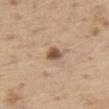Impression: No biopsy was performed on this lesion — it was imaged during a full skin examination and was not determined to be concerning. Clinical summary: Captured under white-light illumination. Cropped from a whole-body photographic skin survey; the tile spans about 15 mm. From the left thigh. Automated image analysis of the tile measured a footprint of about 4 mm², a shape eccentricity near 0.7, and a symmetry-axis asymmetry near 0.2. And it measured a mean CIELAB color near L≈54 a*≈18 b*≈30, a lesion–skin lightness drop of about 14, and a normalized border contrast of about 9. The subject is a male approximately 70 years of age.